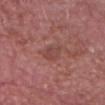Q: Is there a histopathology result?
A: total-body-photography surveillance lesion; no biopsy
Q: How was this image acquired?
A: ~15 mm tile from a whole-body skin photo
Q: Lesion location?
A: the head or neck
Q: Patient demographics?
A: male, roughly 85 years of age or older